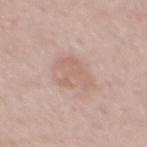Q: Was a biopsy performed?
A: no biopsy performed (imaged during a skin exam)
Q: Where on the body is the lesion?
A: the back
Q: What is the lesion's diameter?
A: ≈4 mm
Q: What did automated image analysis measure?
A: a lesion color around L≈62 a*≈19 b*≈25 in CIELAB, about 7 CIELAB-L* units darker than the surrounding skin, and a lesion-to-skin contrast of about 5 (normalized; higher = more distinct); border irregularity of about 6.5 on a 0–10 scale
Q: Patient demographics?
A: male, in their mid- to late 40s
Q: What lighting was used for the tile?
A: white-light
Q: How was this image acquired?
A: 15 mm crop, total-body photography